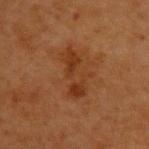{"biopsy_status": "not biopsied; imaged during a skin examination", "lesion_size": {"long_diameter_mm_approx": 5.5}, "lighting": "cross-polarized", "automated_metrics": {"area_mm2_approx": 10.0, "eccentricity": 0.9, "shape_asymmetry": 0.35, "cielab_L": 31, "cielab_a": 22, "cielab_b": 31, "vs_skin_darker_L": 7.0, "vs_skin_contrast_norm": 7.0, "border_irregularity_0_10": 6.0, "color_variation_0_10": 4.0, "lesion_detection_confidence_0_100": 100}, "patient": {"sex": "female", "age_approx": 40}, "site": "upper back", "image": {"source": "total-body photography crop", "field_of_view_mm": 15}}